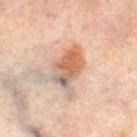notes — imaged on a skin check; not biopsied | location — the right thigh | lesion diameter — about 6 mm | imaging modality — 15 mm crop, total-body photography | subject — female, aged around 50.